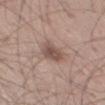Case summary:
* biopsy status — imaged on a skin check; not biopsied
* illumination — white-light
* automated lesion analysis — a footprint of about 6 mm², an outline eccentricity of about 0.8 (0 = round, 1 = elongated), and a symmetry-axis asymmetry near 0.3; a lesion color around L≈51 a*≈17 b*≈23 in CIELAB and a lesion–skin lightness drop of about 11; a classifier nevus-likeness of about 40/100 and lesion-presence confidence of about 100/100
* site — the left thigh
* subject — male, roughly 60 years of age
* image — ~15 mm tile from a whole-body skin photo
* lesion size — ~3.5 mm (longest diameter)